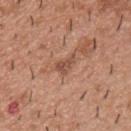Clinical summary: Automated image analysis of the tile measured an average lesion color of about L≈51 a*≈22 b*≈29 (CIELAB), a lesion–skin lightness drop of about 9, and a lesion-to-skin contrast of about 6.5 (normalized; higher = more distinct). And it measured an automated nevus-likeness rating near 0 out of 100 and lesion-presence confidence of about 100/100. From the upper back. The lesion's longest dimension is about 3 mm. Captured under white-light illumination. A male patient, roughly 40 years of age. A region of skin cropped from a whole-body photographic capture, roughly 15 mm wide.